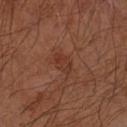Background:
From the left forearm. The lesion's longest dimension is about 2.5 mm. A roughly 15 mm field-of-view crop from a total-body skin photograph. The subject is a male about 65 years old. This is a cross-polarized tile.